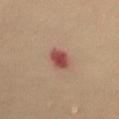follow-up = total-body-photography surveillance lesion; no biopsy | image = ~15 mm tile from a whole-body skin photo | subject = female, aged around 55 | body site = the upper back.